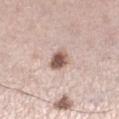site: the leg
lighting: white-light illumination
patient: female, about 45 years old
TBP lesion metrics: an average lesion color of about L≈57 a*≈18 b*≈24 (CIELAB) and a lesion–skin lightness drop of about 16; a border-irregularity index near 2/10, internal color variation of about 4.5 on a 0–10 scale, and radial color variation of about 1.5
diameter: about 2.5 mm
imaging modality: ~15 mm crop, total-body skin-cancer survey